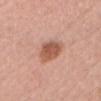Captured during whole-body skin photography for melanoma surveillance; the lesion was not biopsied.
A female subject, in their 50s.
The lesion is located on the head or neck.
Cropped from a total-body skin-imaging series; the visible field is about 15 mm.